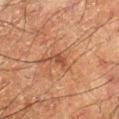Acquisition and patient details:
Automated image analysis of the tile measured about 7 CIELAB-L* units darker than the surrounding skin and a lesion-to-skin contrast of about 6.5 (normalized; higher = more distinct). And it measured a border-irregularity rating of about 3.5/10 and radial color variation of about 0. It also reported a classifier nevus-likeness of about 5/100 and a lesion-detection confidence of about 95/100. On the left forearm. A 15 mm close-up extracted from a 3D total-body photography capture. The subject is a male in their 60s. Approximately 2.5 mm at its widest.